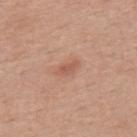Clinical impression:
Captured during whole-body skin photography for melanoma surveillance; the lesion was not biopsied.
Image and clinical context:
Cropped from a total-body skin-imaging series; the visible field is about 15 mm. The total-body-photography lesion software estimated a border-irregularity index near 3/10, a within-lesion color-variation index near 1.5/10, and radial color variation of about 0.5. The recorded lesion diameter is about 2.5 mm. From the mid back. The tile uses white-light illumination. The subject is a male approximately 55 years of age.